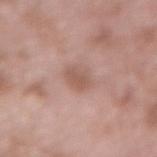follow-up: imaged on a skin check; not biopsied | imaging modality: total-body-photography crop, ~15 mm field of view | site: the lower back | subject: male, roughly 55 years of age.